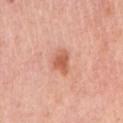Impression:
This lesion was catalogued during total-body skin photography and was not selected for biopsy.
Background:
An algorithmic analysis of the crop reported a footprint of about 5 mm², a shape eccentricity near 0.5, and a shape-asymmetry score of about 0.35 (0 = symmetric). It also reported a border-irregularity index near 3/10, a color-variation rating of about 4/10, and peripheral color asymmetry of about 1.5. Imaged with white-light lighting. A female subject, about 65 years old. The lesion is located on the right upper arm. A region of skin cropped from a whole-body photographic capture, roughly 15 mm wide. Longest diameter approximately 2.5 mm.